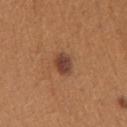Background:
This is a white-light tile. About 2.5 mm across. Automated tile analysis of the lesion measured a footprint of about 5 mm², an eccentricity of roughly 0.4, and a shape-asymmetry score of about 0.2 (0 = symmetric). It also reported a mean CIELAB color near L≈42 a*≈22 b*≈29 and a lesion-to-skin contrast of about 10 (normalized; higher = more distinct). A female subject, in their mid- to late 20s. On the left upper arm. A close-up tile cropped from a whole-body skin photograph, about 15 mm across.